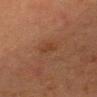Notes:
– notes — total-body-photography surveillance lesion; no biopsy
– automated lesion analysis — a footprint of about 3 mm², a shape eccentricity near 0.8, and two-axis asymmetry of about 0.3; about 5 CIELAB-L* units darker than the surrounding skin and a lesion-to-skin contrast of about 5.5 (normalized; higher = more distinct); a border-irregularity index near 3/10, internal color variation of about 1 on a 0–10 scale, and radial color variation of about 0.5; a lesion-detection confidence of about 100/100
– anatomic site — the head or neck
– imaging modality — ~15 mm tile from a whole-body skin photo
– size — ~2.5 mm (longest diameter)
– patient — male, about 45 years old
– tile lighting — cross-polarized